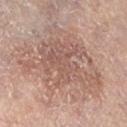Clinical impression:
The lesion was tiled from a total-body skin photograph and was not biopsied.
Acquisition and patient details:
From the left lower leg. Captured under white-light illumination. A female patient in their mid-60s. A lesion tile, about 15 mm wide, cut from a 3D total-body photograph.